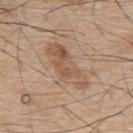Impression: The lesion was photographed on a routine skin check and not biopsied; there is no pathology result. Image and clinical context: Located on the back. A 15 mm close-up tile from a total-body photography series done for melanoma screening. A male subject, approximately 75 years of age. Approximately 7 mm at its widest. Automated tile analysis of the lesion measured a normalized lesion–skin contrast near 6.5. And it measured border irregularity of about 3.5 on a 0–10 scale, a color-variation rating of about 5.5/10, and radial color variation of about 2. It also reported an automated nevus-likeness rating near 0 out of 100 and a detector confidence of about 100 out of 100 that the crop contains a lesion. Imaged with white-light lighting.